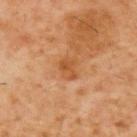The lesion is on the upper back. A close-up tile cropped from a whole-body skin photograph, about 15 mm across. The patient is a male in their 60s.